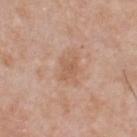This lesion was catalogued during total-body skin photography and was not selected for biopsy.
This is a white-light tile.
Longest diameter approximately 2.5 mm.
A lesion tile, about 15 mm wide, cut from a 3D total-body photograph.
A male patient, about 50 years old.
The lesion is on the chest.